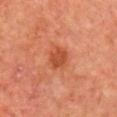{
  "biopsy_status": "not biopsied; imaged during a skin examination",
  "lesion_size": {
    "long_diameter_mm_approx": 3.0
  },
  "image": {
    "source": "total-body photography crop",
    "field_of_view_mm": 15
  },
  "patient": {
    "sex": "male",
    "age_approx": 65
  },
  "lighting": "cross-polarized",
  "site": "right upper arm"
}